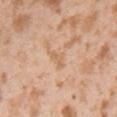Notes:
* image-analysis metrics: an area of roughly 3.5 mm², an outline eccentricity of about 0.75 (0 = round, 1 = elongated), and a symmetry-axis asymmetry near 0.4; a border-irregularity index near 4/10, a within-lesion color-variation index near 0.5/10, and peripheral color asymmetry of about 0; a lesion-detection confidence of about 100/100
* lesion diameter: ~2.5 mm (longest diameter)
* image: 15 mm crop, total-body photography
* lighting: white-light illumination
* subject: male, aged 23 to 27
* location: the left upper arm